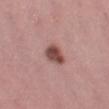site = the left thigh; subject = female, about 45 years old; diameter = ~3 mm (longest diameter); imaging modality = 15 mm crop, total-body photography; lighting = white-light illumination.